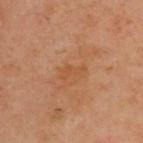Findings:
– workup — no biopsy performed (imaged during a skin exam)